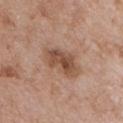The lesion is on the chest. The subject is a male in their mid-60s. A close-up tile cropped from a whole-body skin photograph, about 15 mm across.